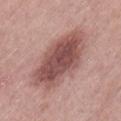site = the left thigh; image source = ~15 mm crop, total-body skin-cancer survey; patient = female, approximately 40 years of age.